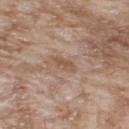Clinical impression:
No biopsy was performed on this lesion — it was imaged during a full skin examination and was not determined to be concerning.
Acquisition and patient details:
A 15 mm close-up extracted from a 3D total-body photography capture. The patient is a male roughly 65 years of age. This is a white-light tile. Automated image analysis of the tile measured a mean CIELAB color near L≈53 a*≈17 b*≈28 and a normalized lesion–skin contrast near 6. The software also gave a color-variation rating of about 0/10 and radial color variation of about 0. The recorded lesion diameter is about 3 mm. Located on the upper back.